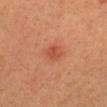Clinical impression:
The lesion was photographed on a routine skin check and not biopsied; there is no pathology result.
Clinical summary:
From the head or neck. The subject is a male roughly 40 years of age. A lesion tile, about 15 mm wide, cut from a 3D total-body photograph. Captured under cross-polarized illumination.